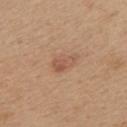Assessment: Recorded during total-body skin imaging; not selected for excision or biopsy. Background: A close-up tile cropped from a whole-body skin photograph, about 15 mm across. Approximately 3 mm at its widest. The tile uses white-light illumination. The lesion is on the mid back. A female subject roughly 45 years of age.